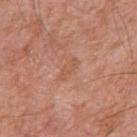Notes:
* notes — no biopsy performed (imaged during a skin exam)
* subject — male, aged 53–57
* image-analysis metrics — a footprint of about 3 mm², a shape eccentricity near 0.9, and a symmetry-axis asymmetry near 0.35; a lesion color around L≈55 a*≈24 b*≈33 in CIELAB, a lesion–skin lightness drop of about 6, and a lesion-to-skin contrast of about 4.5 (normalized; higher = more distinct); an automated nevus-likeness rating near 0 out of 100 and a detector confidence of about 100 out of 100 that the crop contains a lesion
* image source — ~15 mm crop, total-body skin-cancer survey
* site — the right upper arm
* lesion size — ~3 mm (longest diameter)